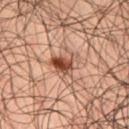The lesion was tiled from a total-body skin photograph and was not biopsied.
The lesion's longest dimension is about 3.5 mm.
On the left thigh.
The lesion-visualizer software estimated a shape eccentricity near 0.75 and a symmetry-axis asymmetry near 0.35.
A 15 mm close-up extracted from a 3D total-body photography capture.
A male patient aged 48 to 52.